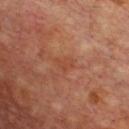{"biopsy_status": "not biopsied; imaged during a skin examination", "lighting": "cross-polarized", "site": "back", "image": {"source": "total-body photography crop", "field_of_view_mm": 15}, "patient": {"sex": "male", "age_approx": 65}}